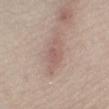No biopsy was performed on this lesion — it was imaged during a full skin examination and was not determined to be concerning. The lesion is on the right lower leg. A female subject aged approximately 60. A 15 mm close-up tile from a total-body photography series done for melanoma screening.